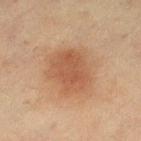This is a cross-polarized tile.
On the left lower leg.
Cropped from a total-body skin-imaging series; the visible field is about 15 mm.
The subject is a female approximately 55 years of age.